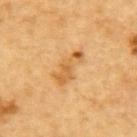Assessment: No biopsy was performed on this lesion — it was imaged during a full skin examination and was not determined to be concerning. Image and clinical context: Approximately 4.5 mm at its widest. Cropped from a whole-body photographic skin survey; the tile spans about 15 mm. Captured under cross-polarized illumination. Located on the upper back. A male subject roughly 85 years of age or older.